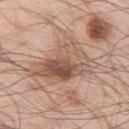Captured during whole-body skin photography for melanoma surveillance; the lesion was not biopsied.
On the left thigh.
A lesion tile, about 15 mm wide, cut from a 3D total-body photograph.
This is a white-light tile.
A male patient aged 53–57.